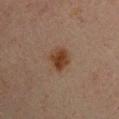<lesion>
<site>left upper arm</site>
<patient>
  <sex>male</sex>
  <age_approx>30</age_approx>
</patient>
<image>
  <source>total-body photography crop</source>
  <field_of_view_mm>15</field_of_view_mm>
</image>
</lesion>